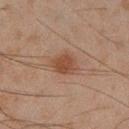{"biopsy_status": "not biopsied; imaged during a skin examination", "patient": {"sex": "male", "age_approx": 45}, "automated_metrics": {"cielab_L": 37, "cielab_a": 18, "cielab_b": 25, "vs_skin_darker_L": 8.0, "vs_skin_contrast_norm": 7.5, "border_irregularity_0_10": 2.0, "color_variation_0_10": 2.0, "peripheral_color_asymmetry": 1.0}, "site": "left lower leg", "lighting": "cross-polarized", "image": {"source": "total-body photography crop", "field_of_view_mm": 15}}